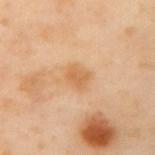<case>
  <biopsy_status>not biopsied; imaged during a skin examination</biopsy_status>
  <site>mid back</site>
  <lighting>cross-polarized</lighting>
  <automated_metrics>
    <cielab_L>65</cielab_L>
    <cielab_a>22</cielab_a>
    <cielab_b>42</cielab_b>
    <vs_skin_contrast_norm>6.5</vs_skin_contrast_norm>
    <nevus_likeness_0_100>10</nevus_likeness_0_100>
    <lesion_detection_confidence_0_100>100</lesion_detection_confidence_0_100>
  </automated_metrics>
  <lesion_size>
    <long_diameter_mm_approx>2.5</long_diameter_mm_approx>
  </lesion_size>
  <image>
    <source>total-body photography crop</source>
    <field_of_view_mm>15</field_of_view_mm>
  </image>
  <patient>
    <sex>female</sex>
    <age_approx>55</age_approx>
  </patient>
</case>